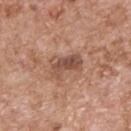Q: Was a biopsy performed?
A: imaged on a skin check; not biopsied
Q: What lighting was used for the tile?
A: white-light illumination
Q: Lesion location?
A: the upper back
Q: What is the imaging modality?
A: ~15 mm tile from a whole-body skin photo
Q: How large is the lesion?
A: ~3.5 mm (longest diameter)
Q: Patient demographics?
A: male, roughly 60 years of age
Q: What did automated image analysis measure?
A: a border-irregularity rating of about 4/10